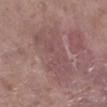* workup · imaged on a skin check; not biopsied
* location · the left lower leg
* TBP lesion metrics · an average lesion color of about L≈50 a*≈20 b*≈19 (CIELAB), about 6 CIELAB-L* units darker than the surrounding skin, and a normalized lesion–skin contrast near 4.5; a nevus-likeness score of about 0/100 and a detector confidence of about 90 out of 100 that the crop contains a lesion
* lesion size · about 7.5 mm
* lighting · white-light illumination
* patient · female, aged 83 or over
* image source · total-body-photography crop, ~15 mm field of view